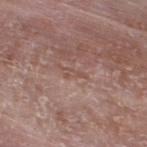Background: A male subject aged approximately 75. A close-up tile cropped from a whole-body skin photograph, about 15 mm across. Located on the leg.